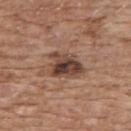The lesion was tiled from a total-body skin photograph and was not biopsied.
The subject is a male aged approximately 60.
A lesion tile, about 15 mm wide, cut from a 3D total-body photograph.
From the chest.